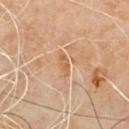Findings:
- biopsy status — total-body-photography surveillance lesion; no biopsy
- imaging modality — ~15 mm tile from a whole-body skin photo
- subject — male, aged approximately 60
- body site — the mid back
- tile lighting — cross-polarized illumination
- automated metrics — a border-irregularity rating of about 3.5/10 and a color-variation rating of about 0/10